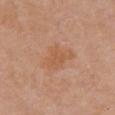workup: total-body-photography surveillance lesion; no biopsy | body site: the chest | patient: female, about 55 years old | imaging modality: 15 mm crop, total-body photography | lighting: white-light illumination | diameter: about 4 mm.